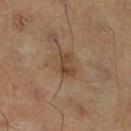<lesion>
<biopsy_status>not biopsied; imaged during a skin examination</biopsy_status>
<lesion_size>
  <long_diameter_mm_approx>3.0</long_diameter_mm_approx>
</lesion_size>
<patient>
  <sex>male</sex>
  <age_approx>45</age_approx>
</patient>
<site>right lower leg</site>
<image>
  <source>total-body photography crop</source>
  <field_of_view_mm>15</field_of_view_mm>
</image>
<lighting>cross-polarized</lighting>
<automated_metrics>
  <cielab_L>43</cielab_L>
  <cielab_a>17</cielab_a>
  <cielab_b>29</cielab_b>
  <vs_skin_darker_L>8.0</vs_skin_darker_L>
  <vs_skin_contrast_norm>7.0</vs_skin_contrast_norm>
  <nevus_likeness_0_100>15</nevus_likeness_0_100>
</automated_metrics>
</lesion>